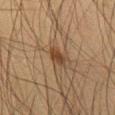image source = ~15 mm crop, total-body skin-cancer survey; illumination = cross-polarized; lesion diameter = about 2.5 mm; anatomic site = the leg; patient = male, in their mid-30s.